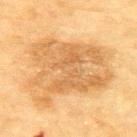No biopsy was performed on this lesion — it was imaged during a full skin examination and was not determined to be concerning. The subject is a female about 80 years old. A lesion tile, about 15 mm wide, cut from a 3D total-body photograph. From the upper back. The lesion's longest dimension is about 9 mm.